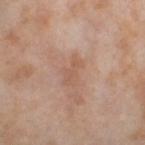notes: total-body-photography surveillance lesion; no biopsy
patient: female, aged approximately 55
image source: total-body-photography crop, ~15 mm field of view
anatomic site: the left thigh